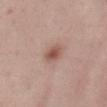Clinical impression:
Captured during whole-body skin photography for melanoma surveillance; the lesion was not biopsied.
Context:
The recorded lesion diameter is about 3 mm. This is a white-light tile. The lesion is located on the abdomen. A male subject, about 55 years old. A close-up tile cropped from a whole-body skin photograph, about 15 mm across.